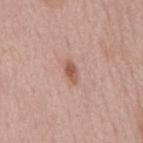Clinical summary: Longest diameter approximately 3 mm. The lesion is on the mid back. The patient is a male aged 53–57. This is a white-light tile. This image is a 15 mm lesion crop taken from a total-body photograph.